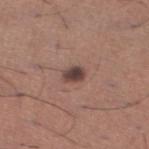Recorded during total-body skin imaging; not selected for excision or biopsy. About 2.5 mm across. Located on the leg. This image is a 15 mm lesion crop taken from a total-body photograph. The lesion-visualizer software estimated a lesion color around L≈41 a*≈18 b*≈20 in CIELAB, about 14 CIELAB-L* units darker than the surrounding skin, and a normalized border contrast of about 11. A male subject, aged 43 to 47. Imaged with white-light lighting.